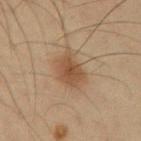– biopsy status — total-body-photography surveillance lesion; no biopsy
– tile lighting — cross-polarized
– subject — male, approximately 35 years of age
– location — the right upper arm
– acquisition — ~15 mm crop, total-body skin-cancer survey